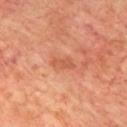biopsy status: no biopsy performed (imaged during a skin exam)
patient: male, about 70 years old
image: ~15 mm crop, total-body skin-cancer survey
anatomic site: the chest